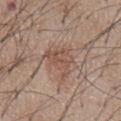Part of a total-body skin-imaging series; this lesion was reviewed on a skin check and was not flagged for biopsy. A male patient, in their mid- to late 50s. The lesion is located on the chest. Longest diameter approximately 4 mm. Cropped from a whole-body photographic skin survey; the tile spans about 15 mm. Captured under white-light illumination. Automated image analysis of the tile measured a lesion color around L≈51 a*≈19 b*≈26 in CIELAB, a lesion–skin lightness drop of about 7, and a normalized lesion–skin contrast near 5.5.